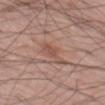Assessment:
Captured during whole-body skin photography for melanoma surveillance; the lesion was not biopsied.
Clinical summary:
A male subject about 60 years old. This image is a 15 mm lesion crop taken from a total-body photograph. Measured at roughly 4.5 mm in maximum diameter. Imaged with white-light lighting. On the left thigh.